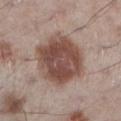The lesion was tiled from a total-body skin photograph and was not biopsied. A 15 mm close-up tile from a total-body photography series done for melanoma screening. Automated tile analysis of the lesion measured a border-irregularity index near 1.5/10, a color-variation rating of about 5/10, and a peripheral color-asymmetry measure near 1.5. Longest diameter approximately 7 mm. This is a white-light tile. The patient is a male approximately 60 years of age. The lesion is on the left lower leg.